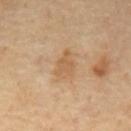No biopsy was performed on this lesion — it was imaged during a full skin examination and was not determined to be concerning.
A 15 mm close-up tile from a total-body photography series done for melanoma screening.
A male subject about 70 years old.
On the mid back.
The total-body-photography lesion software estimated a shape eccentricity near 0.8 and a symmetry-axis asymmetry near 0.3. The software also gave border irregularity of about 4 on a 0–10 scale, internal color variation of about 1.5 on a 0–10 scale, and radial color variation of about 0.5.
The tile uses cross-polarized illumination.
Measured at roughly 3.5 mm in maximum diameter.